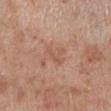biopsy status=no biopsy performed (imaged during a skin exam); acquisition=~15 mm tile from a whole-body skin photo; diameter=~2.5 mm (longest diameter); site=the left lower leg; tile lighting=white-light; patient=male, aged 68–72.